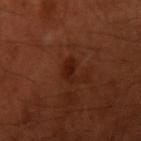The lesion was photographed on a routine skin check and not biopsied; there is no pathology result.